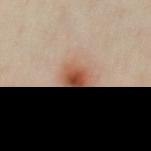Impression:
Part of a total-body skin-imaging series; this lesion was reviewed on a skin check and was not flagged for biopsy.
Image and clinical context:
A male subject in their mid-30s. A lesion tile, about 15 mm wide, cut from a 3D total-body photograph. The lesion is on the front of the torso. Measured at roughly 4 mm in maximum diameter. The total-body-photography lesion software estimated a mean CIELAB color near L≈51 a*≈22 b*≈31, roughly 13 lightness units darker than nearby skin, and a normalized lesion–skin contrast near 10. The analysis additionally found a classifier nevus-likeness of about 100/100 and lesion-presence confidence of about 100/100.